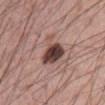Imaged during a routine full-body skin examination; the lesion was not biopsied and no histopathology is available. On the abdomen. Captured under white-light illumination. A male subject, about 55 years old. An algorithmic analysis of the crop reported a lesion area of about 9.5 mm² and an eccentricity of roughly 0.7. And it measured a mean CIELAB color near L≈43 a*≈19 b*≈21 and a lesion–skin lightness drop of about 17. The software also gave border irregularity of about 4.5 on a 0–10 scale and a color-variation rating of about 5/10. It also reported a classifier nevus-likeness of about 100/100. A 15 mm close-up extracted from a 3D total-body photography capture. The lesion's longest dimension is about 4.5 mm.